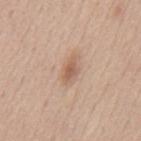Case summary:
- workup · total-body-photography surveillance lesion; no biopsy
- body site · the back
- image · total-body-photography crop, ~15 mm field of view
- patient · male, in their mid-40s